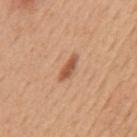<lesion>
  <biopsy_status>not biopsied; imaged during a skin examination</biopsy_status>
  <site>mid back</site>
  <image>
    <source>total-body photography crop</source>
    <field_of_view_mm>15</field_of_view_mm>
  </image>
  <lighting>white-light</lighting>
  <patient>
    <sex>male</sex>
    <age_approx>50</age_approx>
  </patient>
  <lesion_size>
    <long_diameter_mm_approx>3.5</long_diameter_mm_approx>
  </lesion_size>
</lesion>